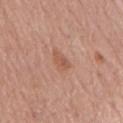Impression: The lesion was photographed on a routine skin check and not biopsied; there is no pathology result. Context: Cropped from a total-body skin-imaging series; the visible field is about 15 mm. From the back. A male subject, approximately 80 years of age.